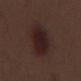Part of a total-body skin-imaging series; this lesion was reviewed on a skin check and was not flagged for biopsy.
Cropped from a whole-body photographic skin survey; the tile spans about 15 mm.
Automated image analysis of the tile measured a border-irregularity rating of about 1.5/10, a color-variation rating of about 4/10, and a peripheral color-asymmetry measure near 1.5. The analysis additionally found a classifier nevus-likeness of about 95/100 and a detector confidence of about 100 out of 100 that the crop contains a lesion.
The recorded lesion diameter is about 6 mm.
A male subject, about 70 years old.
From the right lower leg.